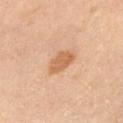This lesion was catalogued during total-body skin photography and was not selected for biopsy.
On the right upper arm.
A 15 mm close-up extracted from a 3D total-body photography capture.
Longest diameter approximately 3.5 mm.
The subject is a female approximately 50 years of age.
Imaged with cross-polarized lighting.